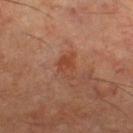Imaged during a routine full-body skin examination; the lesion was not biopsied and no histopathology is available. Automated image analysis of the tile measured a lesion area of about 4.5 mm², an eccentricity of roughly 0.8, and a symmetry-axis asymmetry near 0.35. The analysis additionally found a nevus-likeness score of about 10/100. Measured at roughly 3 mm in maximum diameter. Captured under cross-polarized illumination. Cropped from a total-body skin-imaging series; the visible field is about 15 mm. A male subject, roughly 60 years of age. The lesion is located on the right lower leg.